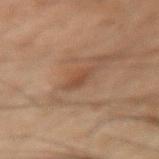Recorded during total-body skin imaging; not selected for excision or biopsy. This image is a 15 mm lesion crop taken from a total-body photograph. The lesion is on the arm. The tile uses cross-polarized illumination. The patient is a male aged 48–52. The total-body-photography lesion software estimated a nevus-likeness score of about 10/100 and lesion-presence confidence of about 90/100.